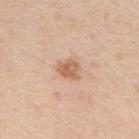Impression:
Captured during whole-body skin photography for melanoma surveillance; the lesion was not biopsied.
Context:
The lesion is located on the upper back. A male subject aged 58–62. A region of skin cropped from a whole-body photographic capture, roughly 15 mm wide. Automated image analysis of the tile measured a lesion area of about 5.5 mm² and a shape eccentricity near 0.6. The software also gave a border-irregularity index near 2/10. And it measured an automated nevus-likeness rating near 55 out of 100.